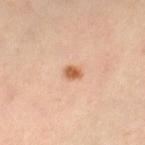Impression:
Imaged during a routine full-body skin examination; the lesion was not biopsied and no histopathology is available.
Background:
The subject is a female aged 38–42. A lesion tile, about 15 mm wide, cut from a 3D total-body photograph. The lesion is located on the right lower leg.